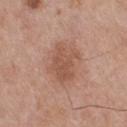A male patient roughly 55 years of age. A roughly 15 mm field-of-view crop from a total-body skin photograph. From the upper back.